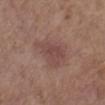No biopsy was performed on this lesion — it was imaged during a full skin examination and was not determined to be concerning. Measured at roughly 5.5 mm in maximum diameter. Located on the left lower leg. A 15 mm crop from a total-body photograph taken for skin-cancer surveillance. Imaged with white-light lighting. The patient is a female approximately 65 years of age.